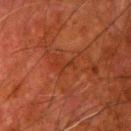Notes:
* body site — the arm
* patient — male, aged around 80
* lighting — cross-polarized
* automated metrics — an eccentricity of roughly 0.9 and a symmetry-axis asymmetry near 0.6; a lesion color around L≈28 a*≈24 b*≈30 in CIELAB, roughly 4 lightness units darker than nearby skin, and a normalized border contrast of about 4.5; an automated nevus-likeness rating near 0 out of 100 and a lesion-detection confidence of about 75/100
* imaging modality — 15 mm crop, total-body photography
* diameter — ~2.5 mm (longest diameter)